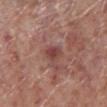Q: Was this lesion biopsied?
A: total-body-photography surveillance lesion; no biopsy
Q: How was this image acquired?
A: 15 mm crop, total-body photography
Q: Patient demographics?
A: male, in their 70s
Q: Where on the body is the lesion?
A: the right lower leg